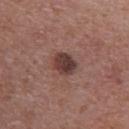Context: The lesion is on the upper back. Approximately 3.5 mm at its widest. A roughly 15 mm field-of-view crop from a total-body skin photograph. The patient is a female aged 58 to 62. Captured under white-light illumination.